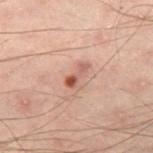Notes:
* biopsy status · catalogued during a skin exam; not biopsied
* lighting · cross-polarized
* subject · male, in their 50s
* site · the left thigh
* imaging modality · ~15 mm crop, total-body skin-cancer survey
* automated metrics · a footprint of about 4 mm², an outline eccentricity of about 0.9 (0 = round, 1 = elongated), and two-axis asymmetry of about 0.5; an average lesion color of about L≈46 a*≈20 b*≈24 (CIELAB) and roughly 9 lightness units darker than nearby skin; a border-irregularity rating of about 5.5/10 and internal color variation of about 3.5 on a 0–10 scale
* diameter · ~3 mm (longest diameter)